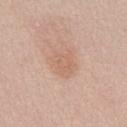No biopsy was performed on this lesion — it was imaged during a full skin examination and was not determined to be concerning. About 3.5 mm across. Captured under white-light illumination. A male patient, aged approximately 55. The lesion is located on the abdomen. Cropped from a total-body skin-imaging series; the visible field is about 15 mm.